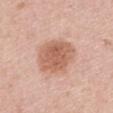Q: What are the patient's age and sex?
A: female, aged 58 to 62
Q: What is the imaging modality?
A: ~15 mm tile from a whole-body skin photo
Q: Lesion location?
A: the back